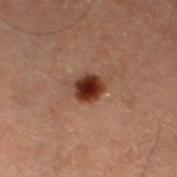Part of a total-body skin-imaging series; this lesion was reviewed on a skin check and was not flagged for biopsy.
On the left thigh.
A male patient about 60 years old.
Captured under cross-polarized illumination.
About 3 mm across.
Cropped from a whole-body photographic skin survey; the tile spans about 15 mm.